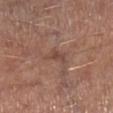Captured during whole-body skin photography for melanoma surveillance; the lesion was not biopsied. Longest diameter approximately 3 mm. Cropped from a total-body skin-imaging series; the visible field is about 15 mm. The lesion is on the right lower leg. A male subject, roughly 65 years of age. Automated tile analysis of the lesion measured a lesion area of about 3 mm², an eccentricity of roughly 0.9, and a symmetry-axis asymmetry near 0.55. It also reported border irregularity of about 5.5 on a 0–10 scale, a within-lesion color-variation index near 1/10, and radial color variation of about 0. And it measured an automated nevus-likeness rating near 0 out of 100 and a detector confidence of about 60 out of 100 that the crop contains a lesion.